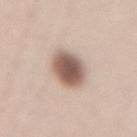Impression: Captured during whole-body skin photography for melanoma surveillance; the lesion was not biopsied. Image and clinical context: A 15 mm close-up tile from a total-body photography series done for melanoma screening. The subject is a female aged around 20. The lesion is located on the lower back.